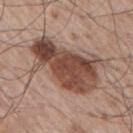Impression: No biopsy was performed on this lesion — it was imaged during a full skin examination and was not determined to be concerning. Clinical summary: A 15 mm close-up tile from a total-body photography series done for melanoma screening. From the right upper arm. The tile uses white-light illumination. About 8.5 mm across. A male patient aged approximately 65.